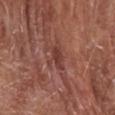biopsy_status: not biopsied; imaged during a skin examination